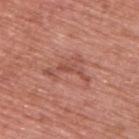No biopsy was performed on this lesion — it was imaged during a full skin examination and was not determined to be concerning. A roughly 15 mm field-of-view crop from a total-body skin photograph. A male patient, aged 68–72. The lesion is on the upper back. Imaged with white-light lighting.